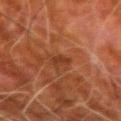Clinical impression:
Captured during whole-body skin photography for melanoma surveillance; the lesion was not biopsied.
Background:
A lesion tile, about 15 mm wide, cut from a 3D total-body photograph. About 2.5 mm across. The tile uses cross-polarized illumination. Automated tile analysis of the lesion measured a lesion area of about 2.5 mm², an outline eccentricity of about 0.9 (0 = round, 1 = elongated), and two-axis asymmetry of about 0.3. It also reported a border-irregularity rating of about 3/10, a within-lesion color-variation index near 1/10, and radial color variation of about 0. The software also gave a lesion-detection confidence of about 100/100. The lesion is on the left upper arm. The subject is a male in their 80s.